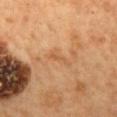Notes:
– biopsy status · imaged on a skin check; not biopsied
– patient · female, approximately 60 years of age
– lesion size · ≈2.5 mm
– body site · the back
– image · 15 mm crop, total-body photography
– illumination · cross-polarized